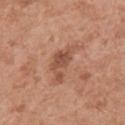{
  "biopsy_status": "not biopsied; imaged during a skin examination",
  "site": "right upper arm",
  "automated_metrics": {
    "cielab_L": 52,
    "cielab_a": 23,
    "cielab_b": 31,
    "vs_skin_contrast_norm": 7.0,
    "border_irregularity_0_10": 6.0,
    "peripheral_color_asymmetry": 1.0
  },
  "lesion_size": {
    "long_diameter_mm_approx": 5.5
  },
  "patient": {
    "sex": "male",
    "age_approx": 55
  },
  "image": {
    "source": "total-body photography crop",
    "field_of_view_mm": 15
  }
}